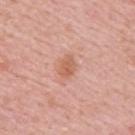Clinical impression:
Captured during whole-body skin photography for melanoma surveillance; the lesion was not biopsied.
Image and clinical context:
The lesion's longest dimension is about 3 mm. The patient is a male roughly 50 years of age. Cropped from a total-body skin-imaging series; the visible field is about 15 mm. The lesion is located on the back.